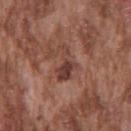| key | value |
|---|---|
| follow-up | catalogued during a skin exam; not biopsied |
| body site | the front of the torso |
| lesion diameter | ≈4.5 mm |
| image | ~15 mm crop, total-body skin-cancer survey |
| subject | male, aged around 75 |
| tile lighting | white-light illumination |
| automated lesion analysis | a lesion–skin lightness drop of about 9 and a normalized lesion–skin contrast near 8; a within-lesion color-variation index near 2.5/10 and peripheral color asymmetry of about 1 |